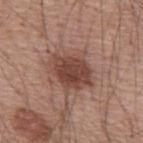Clinical impression:
This lesion was catalogued during total-body skin photography and was not selected for biopsy.
Context:
The lesion is on the back. A close-up tile cropped from a whole-body skin photograph, about 15 mm across. Captured under white-light illumination. The recorded lesion diameter is about 5.5 mm. A male patient, in their mid- to late 50s.